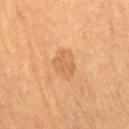{
  "site": "right thigh",
  "image": {
    "source": "total-body photography crop",
    "field_of_view_mm": 15
  },
  "lighting": "cross-polarized",
  "automated_metrics": {
    "area_mm2_approx": 7.5,
    "eccentricity": 0.6,
    "shape_asymmetry": 0.25,
    "nevus_likeness_0_100": 10
  },
  "lesion_size": {
    "long_diameter_mm_approx": 3.5
  },
  "patient": {
    "sex": "female",
    "age_approx": 70
  }
}